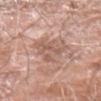Impression: The lesion was photographed on a routine skin check and not biopsied; there is no pathology result. Image and clinical context: From the left forearm. The subject is a male about 60 years old. Approximately 5 mm at its widest. A lesion tile, about 15 mm wide, cut from a 3D total-body photograph. Captured under white-light illumination.